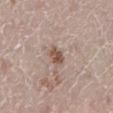biopsy status = imaged on a skin check; not biopsied | anatomic site = the left lower leg | imaging modality = total-body-photography crop, ~15 mm field of view | subject = female, approximately 50 years of age | lesion size = ≈2.5 mm | image-analysis metrics = a shape eccentricity near 0.7 and a symmetry-axis asymmetry near 0.2; a mean CIELAB color near L≈52 a*≈18 b*≈25, about 11 CIELAB-L* units darker than the surrounding skin, and a normalized lesion–skin contrast near 8.5; a color-variation rating of about 3.5/10 and peripheral color asymmetry of about 1; a nevus-likeness score of about 85/100 | tile lighting = white-light.